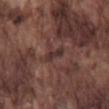A 15 mm close-up tile from a total-body photography series done for melanoma screening.
The lesion-visualizer software estimated a footprint of about 3.5 mm², an eccentricity of roughly 0.9, and two-axis asymmetry of about 0.2. The software also gave an average lesion color of about L≈32 a*≈18 b*≈18 (CIELAB). It also reported border irregularity of about 2.5 on a 0–10 scale, a within-lesion color-variation index near 1.5/10, and a peripheral color-asymmetry measure near 0.5.
From the back.
This is a white-light tile.
Longest diameter approximately 3 mm.
The subject is a male approximately 75 years of age.